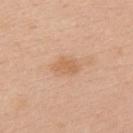{"site": "left upper arm", "lesion_size": {"long_diameter_mm_approx": 3.0}, "image": {"source": "total-body photography crop", "field_of_view_mm": 15}, "patient": {"sex": "female", "age_approx": 35}}